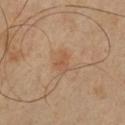biopsy status: catalogued during a skin exam; not biopsied | tile lighting: cross-polarized illumination | size: ~3 mm (longest diameter) | site: the chest | patient: male, aged 38 to 42 | image: 15 mm crop, total-body photography | automated lesion analysis: an average lesion color of about L≈54 a*≈20 b*≈33 (CIELAB) and a normalized border contrast of about 5.5; border irregularity of about 3 on a 0–10 scale, internal color variation of about 2 on a 0–10 scale, and radial color variation of about 0.5; an automated nevus-likeness rating near 5 out of 100 and a detector confidence of about 100 out of 100 that the crop contains a lesion.